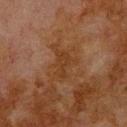Recorded during total-body skin imaging; not selected for excision or biopsy. The lesion is on the upper back. A region of skin cropped from a whole-body photographic capture, roughly 15 mm wide. This is a cross-polarized tile. The patient is a male aged 78 to 82.